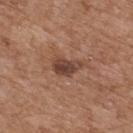Q: Was a biopsy performed?
A: no biopsy performed (imaged during a skin exam)
Q: What are the patient's age and sex?
A: male, about 65 years old
Q: Illumination type?
A: white-light illumination
Q: What kind of image is this?
A: ~15 mm tile from a whole-body skin photo
Q: How large is the lesion?
A: about 3.5 mm
Q: Where on the body is the lesion?
A: the upper back